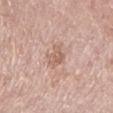<case>
<biopsy_status>not biopsied; imaged during a skin examination</biopsy_status>
<lesion_size>
  <long_diameter_mm_approx>3.0</long_diameter_mm_approx>
</lesion_size>
<image>
  <source>total-body photography crop</source>
  <field_of_view_mm>15</field_of_view_mm>
</image>
<site>left lower leg</site>
<lighting>white-light</lighting>
<patient>
  <sex>female</sex>
  <age_approx>70</age_approx>
</patient>
</case>